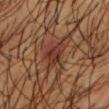No biopsy was performed on this lesion — it was imaged during a full skin examination and was not determined to be concerning. The subject is a male about 50 years old. The lesion is located on the head or neck. A 15 mm crop from a total-body photograph taken for skin-cancer surveillance. Longest diameter approximately 4 mm.